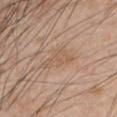Impression:
No biopsy was performed on this lesion — it was imaged during a full skin examination and was not determined to be concerning.
Image and clinical context:
Longest diameter approximately 4.5 mm. A 15 mm close-up tile from a total-body photography series done for melanoma screening. A male patient in their 50s. The lesion is located on the left upper arm. The tile uses white-light illumination.